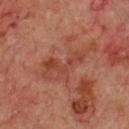biopsy status: catalogued during a skin exam; not biopsied | patient: male, approximately 70 years of age | image source: ~15 mm crop, total-body skin-cancer survey | body site: the chest.